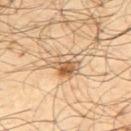Impression:
No biopsy was performed on this lesion — it was imaged during a full skin examination and was not determined to be concerning.
Background:
Measured at roughly 4 mm in maximum diameter. A male subject, approximately 65 years of age. Captured under cross-polarized illumination. Automated tile analysis of the lesion measured a lesion color around L≈59 a*≈17 b*≈34 in CIELAB, a lesion–skin lightness drop of about 11, and a normalized border contrast of about 7.5. It also reported a classifier nevus-likeness of about 95/100 and a lesion-detection confidence of about 100/100. Cropped from a whole-body photographic skin survey; the tile spans about 15 mm. Located on the upper back.